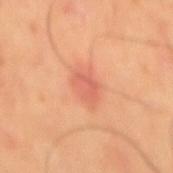The lesion was tiled from a total-body skin photograph and was not biopsied. A male patient, aged 63 to 67. A 15 mm crop from a total-body photograph taken for skin-cancer surveillance. On the back.